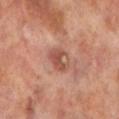Q: Was a biopsy performed?
A: catalogued during a skin exam; not biopsied
Q: Automated lesion metrics?
A: a classifier nevus-likeness of about 0/100 and a lesion-detection confidence of about 100/100
Q: Lesion size?
A: about 3 mm
Q: Lesion location?
A: the left lower leg
Q: Patient demographics?
A: male, approximately 70 years of age
Q: How was this image acquired?
A: total-body-photography crop, ~15 mm field of view